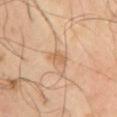{"biopsy_status": "not biopsied; imaged during a skin examination"}